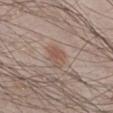Impression:
Captured during whole-body skin photography for melanoma surveillance; the lesion was not biopsied.
Background:
An algorithmic analysis of the crop reported a lesion area of about 5.5 mm², an outline eccentricity of about 0.65 (0 = round, 1 = elongated), and a symmetry-axis asymmetry near 0.25. And it measured a mean CIELAB color near L≈53 a*≈18 b*≈25, roughly 7 lightness units darker than nearby skin, and a normalized lesion–skin contrast near 6. A male subject in their mid- to late 20s. The lesion is located on the right lower leg. Approximately 3 mm at its widest. Captured under white-light illumination. This image is a 15 mm lesion crop taken from a total-body photograph.